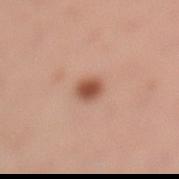Assessment:
The lesion was photographed on a routine skin check and not biopsied; there is no pathology result.
Background:
A female subject roughly 30 years of age. The lesion is located on the left lower leg. A lesion tile, about 15 mm wide, cut from a 3D total-body photograph.